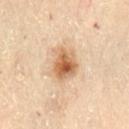follow-up: imaged on a skin check; not biopsied | anatomic site: the lower back | tile lighting: cross-polarized illumination | image: 15 mm crop, total-body photography | automated lesion analysis: an average lesion color of about L≈63 a*≈20 b*≈37 (CIELAB), roughly 14 lightness units darker than nearby skin, and a normalized border contrast of about 9 | subject: female, aged 58 to 62.